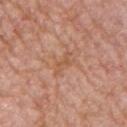Part of a total-body skin-imaging series; this lesion was reviewed on a skin check and was not flagged for biopsy. A male subject, about 55 years old. Automated tile analysis of the lesion measured an average lesion color of about L≈55 a*≈23 b*≈33 (CIELAB), about 6 CIELAB-L* units darker than the surrounding skin, and a normalized lesion–skin contrast near 5.5. The software also gave an automated nevus-likeness rating near 0 out of 100. Located on the left upper arm. A close-up tile cropped from a whole-body skin photograph, about 15 mm across. Approximately 3 mm at its widest.